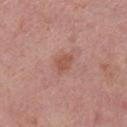biopsy_status: not biopsied; imaged during a skin examination
patient:
  sex: female
  age_approx: 40
site: left lower leg
image:
  source: total-body photography crop
  field_of_view_mm: 15